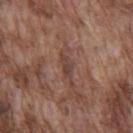Imaged during a routine full-body skin examination; the lesion was not biopsied and no histopathology is available. Cropped from a whole-body photographic skin survey; the tile spans about 15 mm. The recorded lesion diameter is about 3 mm. Automated image analysis of the tile measured an average lesion color of about L≈41 a*≈19 b*≈24 (CIELAB) and roughly 8 lightness units darker than nearby skin. The analysis additionally found a nevus-likeness score of about 0/100 and lesion-presence confidence of about 65/100. A male subject about 75 years old. The tile uses white-light illumination. From the chest.